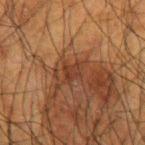{"biopsy_status": "not biopsied; imaged during a skin examination", "site": "right forearm", "image": {"source": "total-body photography crop", "field_of_view_mm": 15}, "lighting": "cross-polarized", "automated_metrics": {"area_mm2_approx": 4.0, "eccentricity": 0.7, "shape_asymmetry": 0.3, "border_irregularity_0_10": 3.0, "color_variation_0_10": 1.5, "peripheral_color_asymmetry": 0.5, "nevus_likeness_0_100": 0, "lesion_detection_confidence_0_100": 80}, "patient": {"sex": "male", "age_approx": 60}}